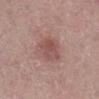biopsy status: no biopsy performed (imaged during a skin exam) | illumination: white-light | image-analysis metrics: a lesion color around L≈50 a*≈22 b*≈22 in CIELAB, roughly 8 lightness units darker than nearby skin, and a normalized lesion–skin contrast near 6; a border-irregularity rating of about 2.5/10 and radial color variation of about 1.5; a lesion-detection confidence of about 100/100 | imaging modality: total-body-photography crop, ~15 mm field of view | lesion size: ≈3 mm | site: the right lower leg | patient: female, in their 40s.